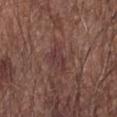<tbp_lesion>
<biopsy_status>not biopsied; imaged during a skin examination</biopsy_status>
<image>
  <source>total-body photography crop</source>
  <field_of_view_mm>15</field_of_view_mm>
</image>
<patient>
  <sex>male</sex>
  <age_approx>65</age_approx>
</patient>
<site>left forearm</site>
<lighting>white-light</lighting>
<automated_metrics>
  <area_mm2_approx>4.5</area_mm2_approx>
  <eccentricity>0.85</eccentricity>
  <shape_asymmetry>0.45</shape_asymmetry>
  <nevus_likeness_0_100>0</nevus_likeness_0_100>
  <lesion_detection_confidence_0_100>90</lesion_detection_confidence_0_100>
</automated_metrics>
<lesion_size>
  <long_diameter_mm_approx>4.0</long_diameter_mm_approx>
</lesion_size>
</tbp_lesion>